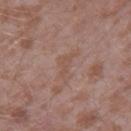The lesion was tiled from a total-body skin photograph and was not biopsied.
From the right thigh.
Approximately 3 mm at its widest.
A 15 mm close-up extracted from a 3D total-body photography capture.
Captured under white-light illumination.
A male subject aged approximately 45.